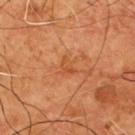follow-up = no biopsy performed (imaged during a skin exam)
automated metrics = a mean CIELAB color near L≈48 a*≈26 b*≈39 and a lesion–skin lightness drop of about 6
acquisition = ~15 mm tile from a whole-body skin photo
lighting = cross-polarized illumination
lesion diameter = ≈2.5 mm
site = the chest
subject = male, aged around 55